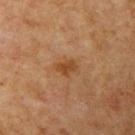Impression: The lesion was tiled from a total-body skin photograph and was not biopsied. Acquisition and patient details: The lesion-visualizer software estimated a mean CIELAB color near L≈41 a*≈20 b*≈34 and a lesion–skin lightness drop of about 7. It also reported border irregularity of about 2.5 on a 0–10 scale, a color-variation rating of about 2.5/10, and peripheral color asymmetry of about 1. A male subject in their 60s. Longest diameter approximately 2.5 mm. A region of skin cropped from a whole-body photographic capture, roughly 15 mm wide. Located on the right upper arm. Captured under cross-polarized illumination.